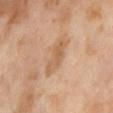Imaged during a routine full-body skin examination; the lesion was not biopsied and no histopathology is available. Located on the abdomen. Captured under cross-polarized illumination. A close-up tile cropped from a whole-body skin photograph, about 15 mm across. The subject is a female roughly 55 years of age. The lesion's longest dimension is about 4.5 mm.